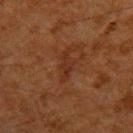The lesion was tiled from a total-body skin photograph and was not biopsied. From the upper back. The tile uses cross-polarized illumination. Measured at roughly 3.5 mm in maximum diameter. The patient is a male aged around 60. A 15 mm close-up extracted from a 3D total-body photography capture.